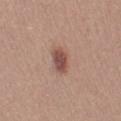Imaged during a routine full-body skin examination; the lesion was not biopsied and no histopathology is available. The lesion is located on the left thigh. Captured under white-light illumination. A female patient, about 25 years old. A close-up tile cropped from a whole-body skin photograph, about 15 mm across. The lesion's longest dimension is about 3.5 mm.